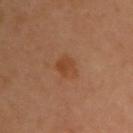Findings:
- workup — imaged on a skin check; not biopsied
- patient — female, roughly 60 years of age
- tile lighting — cross-polarized
- image — ~15 mm tile from a whole-body skin photo
- lesion diameter — ~3 mm (longest diameter)
- body site — the chest